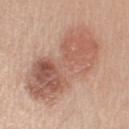Case summary:
* acquisition · ~15 mm tile from a whole-body skin photo
* lighting · white-light illumination
* lesion diameter · ~11.5 mm (longest diameter)
* subject · male, aged around 60
* body site · the arm